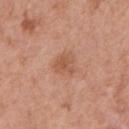This lesion was catalogued during total-body skin photography and was not selected for biopsy.
Imaged with white-light lighting.
A male patient, aged 68 to 72.
A 15 mm close-up tile from a total-body photography series done for melanoma screening.
The total-body-photography lesion software estimated a footprint of about 5 mm², an eccentricity of roughly 0.4, and two-axis asymmetry of about 0.3. The software also gave an average lesion color of about L≈55 a*≈23 b*≈33 (CIELAB), roughly 8 lightness units darker than nearby skin, and a lesion-to-skin contrast of about 6 (normalized; higher = more distinct). The software also gave a border-irregularity rating of about 3.5/10, a within-lesion color-variation index near 2.5/10, and a peripheral color-asymmetry measure near 1. The software also gave a nevus-likeness score of about 10/100 and a detector confidence of about 100 out of 100 that the crop contains a lesion.
On the chest.
Approximately 2.5 mm at its widest.